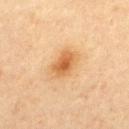Clinical summary:
Located on the upper back. Captured under cross-polarized illumination. A lesion tile, about 15 mm wide, cut from a 3D total-body photograph. The patient is a female aged approximately 45. Approximately 4 mm at its widest.